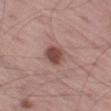Captured during whole-body skin photography for melanoma surveillance; the lesion was not biopsied. The tile uses white-light illumination. A male subject, aged 53–57. From the left thigh. Automated image analysis of the tile measured an area of roughly 8 mm² and an eccentricity of roughly 0.35. And it measured roughly 11 lightness units darker than nearby skin and a normalized border contrast of about 8. The lesion's longest dimension is about 3 mm. A lesion tile, about 15 mm wide, cut from a 3D total-body photograph.